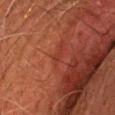This image is a 15 mm lesion crop taken from a total-body photograph. A male patient, aged approximately 65. The lesion is located on the head or neck. Automated tile analysis of the lesion measured about 4 CIELAB-L* units darker than the surrounding skin and a lesion-to-skin contrast of about 4.5 (normalized; higher = more distinct). Captured under cross-polarized illumination.